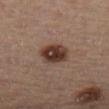  biopsy_status: not biopsied; imaged during a skin examination
  lesion_size:
    long_diameter_mm_approx: 3.5
  automated_metrics:
    area_mm2_approx: 10.0
    eccentricity: 0.5
    shape_asymmetry: 0.15
  site: left leg
  image:
    source: total-body photography crop
    field_of_view_mm: 15
  patient:
    sex: male
    age_approx: 50